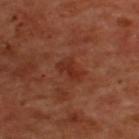The lesion-visualizer software estimated a border-irregularity rating of about 3.5/10, a color-variation rating of about 1/10, and peripheral color asymmetry of about 0.
About 3 mm across.
A 15 mm close-up extracted from a 3D total-body photography capture.
A male subject approximately 50 years of age.
On the upper back.